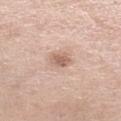  biopsy_status: not biopsied; imaged during a skin examination
  site: left lower leg
  patient:
    sex: female
    age_approx: 55
  image:
    source: total-body photography crop
    field_of_view_mm: 15
  lesion_size:
    long_diameter_mm_approx: 2.5
  lighting: white-light